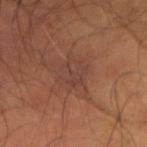{"lesion_size": {"long_diameter_mm_approx": 4.0}, "patient": {"sex": "male", "age_approx": 55}, "site": "arm", "image": {"source": "total-body photography crop", "field_of_view_mm": 15}}